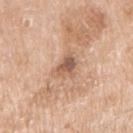Q: Was a biopsy performed?
A: total-body-photography surveillance lesion; no biopsy
Q: What is the anatomic site?
A: the right upper arm
Q: How large is the lesion?
A: ~3 mm (longest diameter)
Q: Patient demographics?
A: male, aged around 65
Q: What kind of image is this?
A: ~15 mm crop, total-body skin-cancer survey